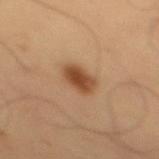The lesion was photographed on a routine skin check and not biopsied; there is no pathology result.
The patient is a male in their mid- to late 50s.
The lesion is located on the mid back.
A lesion tile, about 15 mm wide, cut from a 3D total-body photograph.